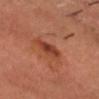Clinical impression:
Captured during whole-body skin photography for melanoma surveillance; the lesion was not biopsied.
Context:
A close-up tile cropped from a whole-body skin photograph, about 15 mm across. On the head or neck. A male subject roughly 65 years of age.